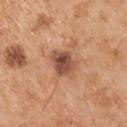Captured during whole-body skin photography for melanoma surveillance; the lesion was not biopsied.
A close-up tile cropped from a whole-body skin photograph, about 15 mm across.
The subject is a male aged 53–57.
Approximately 3.5 mm at its widest.
From the right upper arm.